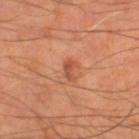Clinical impression:
Part of a total-body skin-imaging series; this lesion was reviewed on a skin check and was not flagged for biopsy.
Context:
Located on the left thigh. A close-up tile cropped from a whole-body skin photograph, about 15 mm across. A male subject, about 70 years old. Imaged with cross-polarized lighting.